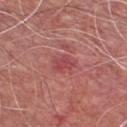notes = no biopsy performed (imaged during a skin exam); imaging modality = ~15 mm tile from a whole-body skin photo; lesion diameter = ≈2.5 mm; site = the chest; subject = male, approximately 60 years of age; illumination = white-light.